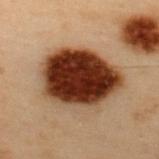Imaged during a routine full-body skin examination; the lesion was not biopsied and no histopathology is available. From the upper back. This is a cross-polarized tile. A male subject, aged around 55. The recorded lesion diameter is about 8.5 mm. A 15 mm close-up tile from a total-body photography series done for melanoma screening.